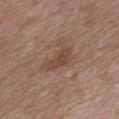Clinical impression:
Captured during whole-body skin photography for melanoma surveillance; the lesion was not biopsied.
Image and clinical context:
The tile uses white-light illumination. A male subject, roughly 50 years of age. This image is a 15 mm lesion crop taken from a total-body photograph. From the front of the torso. The total-body-photography lesion software estimated a border-irregularity index near 4.5/10 and radial color variation of about 0.5. Approximately 4 mm at its widest.